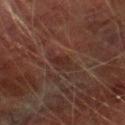Impression: Captured during whole-body skin photography for melanoma surveillance; the lesion was not biopsied. Acquisition and patient details: A male subject, aged approximately 75. This image is a 15 mm lesion crop taken from a total-body photograph. The lesion is located on the right lower leg.